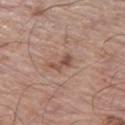biopsy status — catalogued during a skin exam; not biopsied | patient — male, in their mid- to late 60s | imaging modality — ~15 mm tile from a whole-body skin photo | body site — the left thigh.